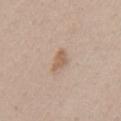Q: Is there a histopathology result?
A: no biopsy performed (imaged during a skin exam)
Q: How large is the lesion?
A: ≈2.5 mm
Q: What are the patient's age and sex?
A: female, aged 38–42
Q: Illumination type?
A: white-light
Q: What is the anatomic site?
A: the arm
Q: What kind of image is this?
A: 15 mm crop, total-body photography